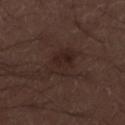The subject is a male in their 30s. The total-body-photography lesion software estimated border irregularity of about 4 on a 0–10 scale, internal color variation of about 3 on a 0–10 scale, and peripheral color asymmetry of about 1. The analysis additionally found a classifier nevus-likeness of about 25/100 and a detector confidence of about 100 out of 100 that the crop contains a lesion. A 15 mm close-up extracted from a 3D total-body photography capture. The lesion's longest dimension is about 4 mm. The lesion is on the right lower leg. Imaged with white-light lighting.